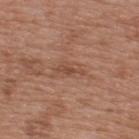Imaged during a routine full-body skin examination; the lesion was not biopsied and no histopathology is available. About 3 mm across. Imaged with white-light lighting. Located on the back. This image is a 15 mm lesion crop taken from a total-body photograph. Automated image analysis of the tile measured a footprint of about 3 mm², an eccentricity of roughly 0.9, and two-axis asymmetry of about 0.5. It also reported border irregularity of about 5.5 on a 0–10 scale, internal color variation of about 0.5 on a 0–10 scale, and a peripheral color-asymmetry measure near 0. The patient is a male aged 48–52.